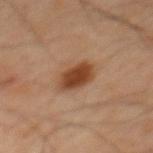Impression: No biopsy was performed on this lesion — it was imaged during a full skin examination and was not determined to be concerning.